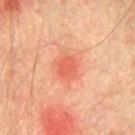follow-up: total-body-photography surveillance lesion; no biopsy
acquisition: total-body-photography crop, ~15 mm field of view
TBP lesion metrics: an outline eccentricity of about 0.6 (0 = round, 1 = elongated) and two-axis asymmetry of about 0.2; a lesion color around L≈51 a*≈31 b*≈32 in CIELAB, about 8 CIELAB-L* units darker than the surrounding skin, and a lesion-to-skin contrast of about 6 (normalized; higher = more distinct)
lesion size: ≈2.5 mm
body site: the chest
tile lighting: cross-polarized
subject: male, aged approximately 75